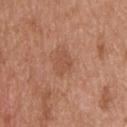No biopsy was performed on this lesion — it was imaged during a full skin examination and was not determined to be concerning. A male subject aged around 60. Located on the upper back. The tile uses white-light illumination. The total-body-photography lesion software estimated an automated nevus-likeness rating near 0 out of 100. This image is a 15 mm lesion crop taken from a total-body photograph. Longest diameter approximately 3 mm.